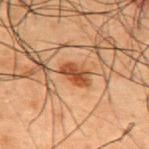The lesion was photographed on a routine skin check and not biopsied; there is no pathology result.
The lesion is located on the upper back.
A male patient in their 50s.
A 15 mm close-up tile from a total-body photography series done for melanoma screening.
The total-body-photography lesion software estimated a footprint of about 3 mm² and a shape-asymmetry score of about 0.35 (0 = symmetric). The analysis additionally found an average lesion color of about L≈35 a*≈22 b*≈31 (CIELAB) and roughly 12 lightness units darker than nearby skin. It also reported a border-irregularity index near 3.5/10 and a peripheral color-asymmetry measure near 0.5.
Imaged with cross-polarized lighting.